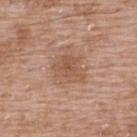Impression:
Recorded during total-body skin imaging; not selected for excision or biopsy.
Context:
The lesion is located on the back. Cropped from a total-body skin-imaging series; the visible field is about 15 mm. A male subject aged 48–52. Imaged with white-light lighting. Longest diameter approximately 4.5 mm.